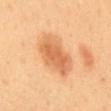No biopsy was performed on this lesion — it was imaged during a full skin examination and was not determined to be concerning. The lesion is located on the back. The patient is a female aged 48–52. A region of skin cropped from a whole-body photographic capture, roughly 15 mm wide. Longest diameter approximately 6.5 mm. Imaged with cross-polarized lighting.